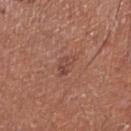No biopsy was performed on this lesion — it was imaged during a full skin examination and was not determined to be concerning. Captured under white-light illumination. Located on the right lower leg. A lesion tile, about 15 mm wide, cut from a 3D total-body photograph. A male subject aged approximately 70.